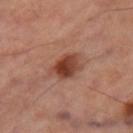Clinical impression: This lesion was catalogued during total-body skin photography and was not selected for biopsy. Background: About 4 mm across. Imaged with cross-polarized lighting. An algorithmic analysis of the crop reported an outline eccentricity of about 0.75 (0 = round, 1 = elongated) and two-axis asymmetry of about 0.25. And it measured a lesion color around L≈43 a*≈25 b*≈30 in CIELAB and roughly 13 lightness units darker than nearby skin. The software also gave border irregularity of about 2.5 on a 0–10 scale and peripheral color asymmetry of about 2. From the leg. A roughly 15 mm field-of-view crop from a total-body skin photograph.